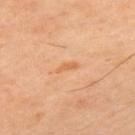biopsy_status: not biopsied; imaged during a skin examination
patient:
  sex: male
  age_approx: 45
lesion_size:
  long_diameter_mm_approx: 2.5
image:
  source: total-body photography crop
  field_of_view_mm: 15
automated_metrics:
  eccentricity: 0.95
  shape_asymmetry: 0.45
  cielab_L: 60
  cielab_a: 24
  cielab_b: 39
  vs_skin_darker_L: 7.0
  vs_skin_contrast_norm: 6.0
  nevus_likeness_0_100: 0
  lesion_detection_confidence_0_100: 100
site: left upper arm